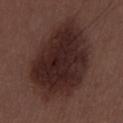workup: imaged on a skin check; not biopsied | acquisition: total-body-photography crop, ~15 mm field of view | automated metrics: an area of roughly 55 mm², an eccentricity of roughly 0.75, and a shape-asymmetry score of about 0.15 (0 = symmetric); a lesion-to-skin contrast of about 11.5 (normalized; higher = more distinct); a border-irregularity index near 2/10, a within-lesion color-variation index near 4.5/10, and a peripheral color-asymmetry measure near 1.5 | site: the mid back | subject: male, roughly 30 years of age | lesion size: ≈10.5 mm.